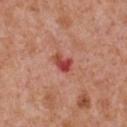Acquisition and patient details:
The subject is a male aged 63–67. This image is a 15 mm lesion crop taken from a total-body photograph. The lesion is located on the chest.